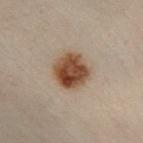biopsy status=imaged on a skin check; not biopsied
TBP lesion metrics=an area of roughly 12 mm², an eccentricity of roughly 0.15, and two-axis asymmetry of about 0.1; border irregularity of about 1 on a 0–10 scale, a within-lesion color-variation index near 5.5/10, and radial color variation of about 2
imaging modality=15 mm crop, total-body photography
patient=male, aged 48–52
illumination=cross-polarized
site=the left leg